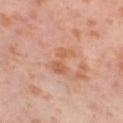biopsy status: total-body-photography surveillance lesion; no biopsy | body site: the right thigh | image source: ~15 mm tile from a whole-body skin photo | illumination: cross-polarized illumination | subject: female, about 55 years old | lesion diameter: ≈4 mm.